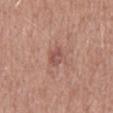Captured during whole-body skin photography for melanoma surveillance; the lesion was not biopsied.
A male patient aged approximately 50.
About 3 mm across.
The total-body-photography lesion software estimated a mean CIELAB color near L≈52 a*≈24 b*≈24, a lesion–skin lightness drop of about 9, and a normalized lesion–skin contrast near 6.5.
This is a white-light tile.
On the mid back.
A 15 mm close-up extracted from a 3D total-body photography capture.